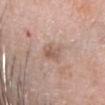No biopsy was performed on this lesion — it was imaged during a full skin examination and was not determined to be concerning. The subject is a female about 60 years old. The lesion is on the head or neck. Cropped from a whole-body photographic skin survey; the tile spans about 15 mm.